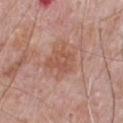biopsy_status: not biopsied; imaged during a skin examination
lesion_size:
  long_diameter_mm_approx: 3.5
image:
  source: total-body photography crop
  field_of_view_mm: 15
patient:
  sex: male
  age_approx: 70
automated_metrics:
  border_irregularity_0_10: 3.5
  color_variation_0_10: 2.0
  peripheral_color_asymmetry: 0.5
  nevus_likeness_0_100: 0
  lesion_detection_confidence_0_100: 100
site: chest